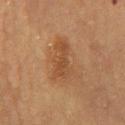lighting: cross-polarized illumination; body site: the front of the torso; image: ~15 mm crop, total-body skin-cancer survey; subject: female, aged approximately 60; size: ≈6 mm.